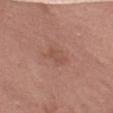follow-up = no biopsy performed (imaged during a skin exam) | imaging modality = total-body-photography crop, ~15 mm field of view | patient = female, aged 38–42 | anatomic site = the abdomen | diameter = ~2.5 mm (longest diameter) | automated metrics = about 6 CIELAB-L* units darker than the surrounding skin and a normalized lesion–skin contrast near 4.5; border irregularity of about 5 on a 0–10 scale and a within-lesion color-variation index near 0/10.